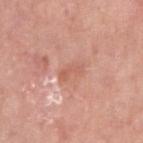Q: Is there a histopathology result?
A: imaged on a skin check; not biopsied
Q: How large is the lesion?
A: about 3 mm
Q: Where on the body is the lesion?
A: the right upper arm
Q: Illumination type?
A: white-light
Q: What is the imaging modality?
A: ~15 mm crop, total-body skin-cancer survey
Q: Who is the patient?
A: female, aged 63–67